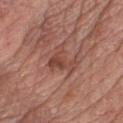| feature | finding |
|---|---|
| biopsy status | imaged on a skin check; not biopsied |